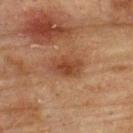No biopsy was performed on this lesion — it was imaged during a full skin examination and was not determined to be concerning.
A male subject in their mid- to late 70s.
A 15 mm crop from a total-body photograph taken for skin-cancer surveillance.
The lesion is located on the upper back.
The lesion's longest dimension is about 3.5 mm.
The tile uses cross-polarized illumination.